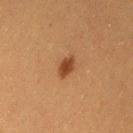* workup: total-body-photography surveillance lesion; no biopsy
* site: the left thigh
* image source: ~15 mm crop, total-body skin-cancer survey
* illumination: cross-polarized
* patient: female, aged 38 to 42
* automated metrics: a mean CIELAB color near L≈36 a*≈21 b*≈31, roughly 10 lightness units darker than nearby skin, and a normalized lesion–skin contrast near 9; a classifier nevus-likeness of about 100/100 and a lesion-detection confidence of about 100/100